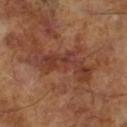Impression:
No biopsy was performed on this lesion — it was imaged during a full skin examination and was not determined to be concerning.
Clinical summary:
The lesion's longest dimension is about 8 mm. A male subject in their mid- to late 60s. Cropped from a total-body skin-imaging series; the visible field is about 15 mm.